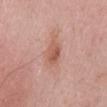No biopsy was performed on this lesion — it was imaged during a full skin examination and was not determined to be concerning. The subject is a male aged 68–72. The total-body-photography lesion software estimated a footprint of about 5 mm², an eccentricity of roughly 0.75, and a shape-asymmetry score of about 0.2 (0 = symmetric). The analysis additionally found a border-irregularity index near 2/10, a within-lesion color-variation index near 4.5/10, and a peripheral color-asymmetry measure near 1.5. It also reported a classifier nevus-likeness of about 30/100 and lesion-presence confidence of about 100/100. Measured at roughly 3 mm in maximum diameter. The lesion is on the back. Imaged with white-light lighting. A 15 mm close-up tile from a total-body photography series done for melanoma screening.